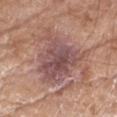Impression: No biopsy was performed on this lesion — it was imaged during a full skin examination and was not determined to be concerning. Context: Captured under white-light illumination. Cropped from a total-body skin-imaging series; the visible field is about 15 mm. A male patient, aged 78 to 82. Located on the right forearm.